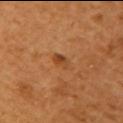Impression: The lesion was photographed on a routine skin check and not biopsied; there is no pathology result. Clinical summary: Measured at roughly 2 mm in maximum diameter. Imaged with cross-polarized lighting. The patient is a female aged approximately 55. On the upper back. Automated tile analysis of the lesion measured a lesion area of about 3 mm², an eccentricity of roughly 0.8, and a symmetry-axis asymmetry near 0.2. And it measured a within-lesion color-variation index near 4/10 and a peripheral color-asymmetry measure near 1.5. The analysis additionally found a nevus-likeness score of about 95/100 and a detector confidence of about 100 out of 100 that the crop contains a lesion. A 15 mm crop from a total-body photograph taken for skin-cancer surveillance.